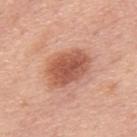Findings:
• follow-up: no biopsy performed (imaged during a skin exam)
• body site: the mid back
• size: about 5.5 mm
• automated metrics: a lesion area of about 15 mm² and an eccentricity of roughly 0.75; a border-irregularity index near 2/10, a within-lesion color-variation index near 4/10, and a peripheral color-asymmetry measure near 1; an automated nevus-likeness rating near 100 out of 100 and a detector confidence of about 100 out of 100 that the crop contains a lesion
• illumination: white-light
• image: ~15 mm tile from a whole-body skin photo
• subject: male, in their mid- to late 70s